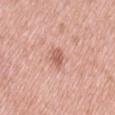Imaged during a routine full-body skin examination; the lesion was not biopsied and no histopathology is available. The lesion is on the leg. A close-up tile cropped from a whole-body skin photograph, about 15 mm across. The tile uses white-light illumination. The patient is a female in their mid- to late 50s.